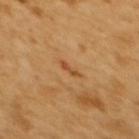{"biopsy_status": "not biopsied; imaged during a skin examination", "patient": {"sex": "female", "age_approx": 55}, "image": {"source": "total-body photography crop", "field_of_view_mm": 15}, "lesion_size": {"long_diameter_mm_approx": 2.5}, "site": "upper back", "lighting": "cross-polarized"}